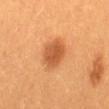No biopsy was performed on this lesion — it was imaged during a full skin examination and was not determined to be concerning. On the mid back. A 15 mm close-up tile from a total-body photography series done for melanoma screening. The subject is a female roughly 30 years of age.